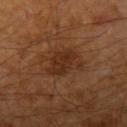| feature | finding |
|---|---|
| workup | catalogued during a skin exam; not biopsied |
| subject | male, roughly 60 years of age |
| lesion diameter | about 4 mm |
| image | total-body-photography crop, ~15 mm field of view |
| image-analysis metrics | a border-irregularity rating of about 3.5/10 and internal color variation of about 2 on a 0–10 scale |
| location | the left upper arm |
| tile lighting | cross-polarized |